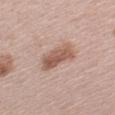| key | value |
|---|---|
| biopsy status | catalogued during a skin exam; not biopsied |
| patient | female, aged approximately 45 |
| illumination | white-light illumination |
| body site | the left upper arm |
| image | 15 mm crop, total-body photography |
| diameter | about 4.5 mm |
| TBP lesion metrics | an average lesion color of about L≈56 a*≈20 b*≈27 (CIELAB), roughly 12 lightness units darker than nearby skin, and a normalized border contrast of about 8; internal color variation of about 4 on a 0–10 scale and peripheral color asymmetry of about 1.5 |